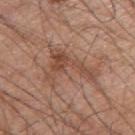This lesion was catalogued during total-body skin photography and was not selected for biopsy. From the right upper arm. A male subject, in their mid-60s. A lesion tile, about 15 mm wide, cut from a 3D total-body photograph.